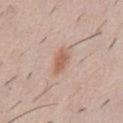Part of a total-body skin-imaging series; this lesion was reviewed on a skin check and was not flagged for biopsy.
This image is a 15 mm lesion crop taken from a total-body photograph.
From the abdomen.
A male subject, aged 48 to 52.